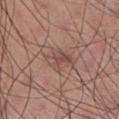* workup — catalogued during a skin exam; not biopsied
* image source — ~15 mm crop, total-body skin-cancer survey
* patient — male, about 45 years old
* body site — the right lower leg